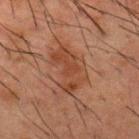Recorded during total-body skin imaging; not selected for excision or biopsy. Automated tile analysis of the lesion measured a shape eccentricity near 0.8 and a symmetry-axis asymmetry near 0.4. The analysis additionally found a border-irregularity index near 5/10, a within-lesion color-variation index near 3/10, and radial color variation of about 1. And it measured a detector confidence of about 100 out of 100 that the crop contains a lesion. Cropped from a whole-body photographic skin survey; the tile spans about 15 mm. The lesion is located on the upper back. Measured at roughly 5.5 mm in maximum diameter. The tile uses cross-polarized illumination. The subject is a male roughly 50 years of age.